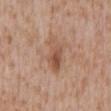| field | value |
|---|---|
| image | total-body-photography crop, ~15 mm field of view |
| subject | male, in their mid- to late 40s |
| anatomic site | the chest |